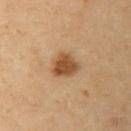Clinical impression:
Part of a total-body skin-imaging series; this lesion was reviewed on a skin check and was not flagged for biopsy.
Acquisition and patient details:
A female subject, aged around 40. Located on the arm. Measured at roughly 3.5 mm in maximum diameter. A 15 mm close-up extracted from a 3D total-body photography capture.